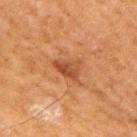Clinical impression: The lesion was tiled from a total-body skin photograph and was not biopsied. Image and clinical context: A male subject, roughly 65 years of age. The total-body-photography lesion software estimated a footprint of about 6 mm², an outline eccentricity of about 0.8 (0 = round, 1 = elongated), and a shape-asymmetry score of about 0.4 (0 = symmetric). The software also gave a mean CIELAB color near L≈43 a*≈24 b*≈35, about 9 CIELAB-L* units darker than the surrounding skin, and a normalized border contrast of about 7. The software also gave a border-irregularity index near 4/10, a color-variation rating of about 3.5/10, and a peripheral color-asymmetry measure near 1.5. The software also gave an automated nevus-likeness rating near 35 out of 100 and a lesion-detection confidence of about 100/100. On the right upper arm. A 15 mm crop from a total-body photograph taken for skin-cancer surveillance.